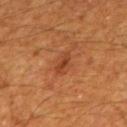diameter = about 3 mm | subject = male, roughly 60 years of age | location = the back | illumination = cross-polarized | image = ~15 mm tile from a whole-body skin photo.